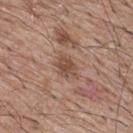{
  "lighting": "white-light",
  "image": {
    "source": "total-body photography crop",
    "field_of_view_mm": 15
  },
  "lesion_size": {
    "long_diameter_mm_approx": 3.0
  },
  "site": "upper back",
  "automated_metrics": {
    "area_mm2_approx": 5.5,
    "border_irregularity_0_10": 2.5,
    "color_variation_0_10": 2.5,
    "peripheral_color_asymmetry": 1.0
  },
  "patient": {
    "sex": "male",
    "age_approx": 65
  }
}